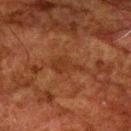This is a cross-polarized tile.
The patient is a male aged around 80.
This image is a 15 mm lesion crop taken from a total-body photograph.
Automated tile analysis of the lesion measured an outline eccentricity of about 0.9 (0 = round, 1 = elongated). And it measured an average lesion color of about L≈27 a*≈21 b*≈28 (CIELAB), roughly 5 lightness units darker than nearby skin, and a normalized lesion–skin contrast near 5.5. The analysis additionally found a border-irregularity rating of about 5.5/10, a within-lesion color-variation index near 1.5/10, and peripheral color asymmetry of about 0.5.
On the arm.
Measured at roughly 3.5 mm in maximum diameter.